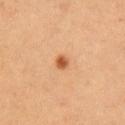workup: imaged on a skin check; not biopsied | patient: female, about 30 years old | image: ~15 mm crop, total-body skin-cancer survey | anatomic site: the right upper arm | lighting: cross-polarized | automated lesion analysis: a within-lesion color-variation index near 1.5/10 | diameter: ~2 mm (longest diameter).